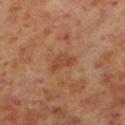Q: Was a biopsy performed?
A: no biopsy performed (imaged during a skin exam)
Q: What is the anatomic site?
A: the left lower leg
Q: What is the imaging modality?
A: 15 mm crop, total-body photography
Q: What is the lesion's diameter?
A: ≈3 mm
Q: Patient demographics?
A: male, aged 58 to 62
Q: Illumination type?
A: cross-polarized